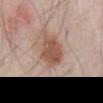This lesion was catalogued during total-body skin photography and was not selected for biopsy.
On the chest.
A 15 mm close-up extracted from a 3D total-body photography capture.
A male subject aged 68–72.